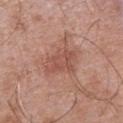biopsy status=no biopsy performed (imaged during a skin exam)
site=the chest
image=~15 mm crop, total-body skin-cancer survey
patient=male, approximately 70 years of age
size=~4 mm (longest diameter)
tile lighting=white-light